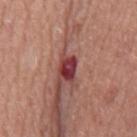{"biopsy_status": "not biopsied; imaged during a skin examination", "site": "mid back", "automated_metrics": {"cielab_L": 38, "cielab_a": 33, "cielab_b": 21, "vs_skin_darker_L": 16.0, "vs_skin_contrast_norm": 13.0, "border_irregularity_0_10": 3.0, "color_variation_0_10": 5.5, "nevus_likeness_0_100": 0, "lesion_detection_confidence_0_100": 100}, "patient": {"sex": "male", "age_approx": 75}, "lesion_size": {"long_diameter_mm_approx": 2.5}, "lighting": "white-light", "image": {"source": "total-body photography crop", "field_of_view_mm": 15}}